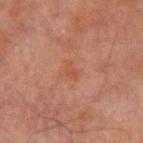notes=catalogued during a skin exam; not biopsied | site=the left upper arm | acquisition=~15 mm crop, total-body skin-cancer survey | subject=male, roughly 60 years of age.